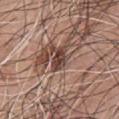The lesion was tiled from a total-body skin photograph and was not biopsied. A male subject, approximately 75 years of age. On the front of the torso. This is a white-light tile. Measured at roughly 3 mm in maximum diameter. A 15 mm crop from a total-body photograph taken for skin-cancer surveillance.